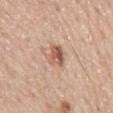Assessment:
Imaged during a routine full-body skin examination; the lesion was not biopsied and no histopathology is available.
Acquisition and patient details:
Imaged with white-light lighting. The recorded lesion diameter is about 3 mm. On the mid back. A male subject aged around 65. This image is a 15 mm lesion crop taken from a total-body photograph.